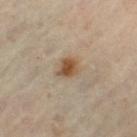The lesion was tiled from a total-body skin photograph and was not biopsied.
A close-up tile cropped from a whole-body skin photograph, about 15 mm across.
The subject is a female aged 58 to 62.
The tile uses cross-polarized illumination.
On the left thigh.